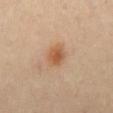Automated tile analysis of the lesion measured a lesion area of about 5.5 mm² and a symmetry-axis asymmetry near 0.2. It also reported a lesion color around L≈54 a*≈21 b*≈34 in CIELAB, about 10 CIELAB-L* units darker than the surrounding skin, and a lesion-to-skin contrast of about 7.5 (normalized; higher = more distinct). The analysis additionally found a nevus-likeness score of about 95/100 and a detector confidence of about 100 out of 100 that the crop contains a lesion.
The lesion is on the lower back.
A roughly 15 mm field-of-view crop from a total-body skin photograph.
Imaged with cross-polarized lighting.
Longest diameter approximately 3 mm.
A male patient, about 55 years old.